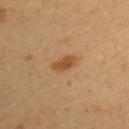notes: total-body-photography surveillance lesion; no biopsy | image source: total-body-photography crop, ~15 mm field of view | patient: female, approximately 40 years of age | image-analysis metrics: a lesion–skin lightness drop of about 10 and a normalized border contrast of about 8; a nevus-likeness score of about 90/100 | illumination: cross-polarized illumination | lesion diameter: about 3 mm | site: the left upper arm.